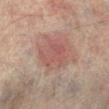{
  "biopsy_status": "not biopsied; imaged during a skin examination",
  "lighting": "cross-polarized",
  "image": {
    "source": "total-body photography crop",
    "field_of_view_mm": 15
  },
  "automated_metrics": {
    "eccentricity": 0.6,
    "shape_asymmetry": 0.2,
    "vs_skin_darker_L": 6.0,
    "vs_skin_contrast_norm": 5.0,
    "nevus_likeness_0_100": 55
  },
  "lesion_size": {
    "long_diameter_mm_approx": 5.0
  },
  "site": "left lower leg",
  "patient": {
    "sex": "male",
    "age_approx": 75
  }
}